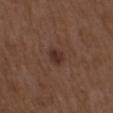site: upper back
patient:
  sex: male
  age_approx: 65
lesion_size:
  long_diameter_mm_approx: 2.5
lighting: white-light
image:
  source: total-body photography crop
  field_of_view_mm: 15
diagnosis:
  histopathology: nodular basal cell carcinoma
  malignancy: malignant
  taxonomic_path:
    - Malignant
    - Malignant adnexal epithelial proliferations - Follicular
    - Basal cell carcinoma
    - Basal cell carcinoma, Nodular